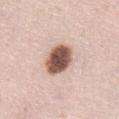Part of a total-body skin-imaging series; this lesion was reviewed on a skin check and was not flagged for biopsy. A female subject, aged around 65. The lesion is located on the abdomen. A region of skin cropped from a whole-body photographic capture, roughly 15 mm wide. The lesion's longest dimension is about 4.5 mm.